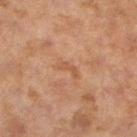| key | value |
|---|---|
| notes | imaged on a skin check; not biopsied |
| imaging modality | total-body-photography crop, ~15 mm field of view |
| location | the left lower leg |
| lighting | cross-polarized |
| patient | female, aged around 60 |
| automated metrics | an outline eccentricity of about 0.9 (0 = round, 1 = elongated) and a shape-asymmetry score of about 0.6 (0 = symmetric); a border-irregularity rating of about 6/10, internal color variation of about 0 on a 0–10 scale, and peripheral color asymmetry of about 0; a nevus-likeness score of about 0/100 |